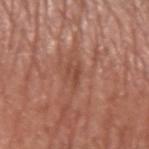<case>
<biopsy_status>not biopsied; imaged during a skin examination</biopsy_status>
<site>arm</site>
<image>
  <source>total-body photography crop</source>
  <field_of_view_mm>15</field_of_view_mm>
</image>
<lesion_size>
  <long_diameter_mm_approx>2.5</long_diameter_mm_approx>
</lesion_size>
<patient>
  <sex>male</sex>
  <age_approx>65</age_approx>
</patient>
</case>